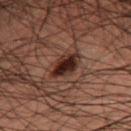Imaged during a routine full-body skin examination; the lesion was not biopsied and no histopathology is available.
On the right thigh.
A roughly 15 mm field-of-view crop from a total-body skin photograph.
The patient is a male aged 48–52.
Automated tile analysis of the lesion measured a footprint of about 5.5 mm², an outline eccentricity of about 0.6 (0 = round, 1 = elongated), and a shape-asymmetry score of about 0.25 (0 = symmetric). The software also gave a lesion color around L≈18 a*≈16 b*≈17 in CIELAB, a lesion–skin lightness drop of about 12, and a lesion-to-skin contrast of about 14.5 (normalized; higher = more distinct). The analysis additionally found lesion-presence confidence of about 100/100.
Approximately 3 mm at its widest.
Captured under cross-polarized illumination.